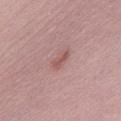<lesion>
  <biopsy_status>not biopsied; imaged during a skin examination</biopsy_status>
  <image>
    <source>total-body photography crop</source>
    <field_of_view_mm>15</field_of_view_mm>
  </image>
  <site>front of the torso</site>
  <patient>
    <sex>male</sex>
    <age_approx>50</age_approx>
  </patient>
</lesion>